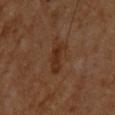follow-up: catalogued during a skin exam; not biopsied
subject: male, aged 58 to 62
image source: total-body-photography crop, ~15 mm field of view
location: the upper back
automated lesion analysis: a footprint of about 5.5 mm² and a symmetry-axis asymmetry near 0.35; a lesion color around L≈29 a*≈20 b*≈29 in CIELAB, about 7 CIELAB-L* units darker than the surrounding skin, and a lesion-to-skin contrast of about 7.5 (normalized; higher = more distinct); lesion-presence confidence of about 100/100
illumination: cross-polarized illumination
diameter: ≈3.5 mm